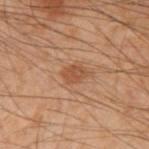Case summary:
- location — the arm
- lesion size — about 2.5 mm
- image — ~15 mm crop, total-body skin-cancer survey
- tile lighting — cross-polarized
- subject — male, aged 48 to 52